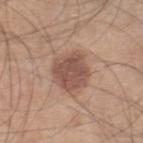Impression: Captured during whole-body skin photography for melanoma surveillance; the lesion was not biopsied. Background: A roughly 15 mm field-of-view crop from a total-body skin photograph. On the right lower leg. The patient is a male aged 43–47. An algorithmic analysis of the crop reported a lesion area of about 13 mm², a shape eccentricity near 0.4, and a shape-asymmetry score of about 0.15 (0 = symmetric). And it measured a nevus-likeness score of about 15/100 and a detector confidence of about 100 out of 100 that the crop contains a lesion. Captured under white-light illumination.